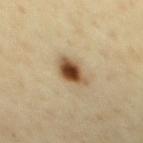<tbp_lesion>
  <biopsy_status>not biopsied; imaged during a skin examination</biopsy_status>
  <lesion_size>
    <long_diameter_mm_approx>3.5</long_diameter_mm_approx>
  </lesion_size>
  <site>chest</site>
  <lighting>cross-polarized</lighting>
  <image>
    <source>total-body photography crop</source>
    <field_of_view_mm>15</field_of_view_mm>
  </image>
  <automated_metrics>
    <area_mm2_approx>7.0</area_mm2_approx>
    <eccentricity>0.65</eccentricity>
    <shape_asymmetry>0.25</shape_asymmetry>
    <vs_skin_darker_L>19.0</vs_skin_darker_L>
    <vs_skin_contrast_norm>13.0</vs_skin_contrast_norm>
    <color_variation_0_10>6.0</color_variation_0_10>
    <peripheral_color_asymmetry>1.0</peripheral_color_asymmetry>
  </automated_metrics>
  <patient>
    <sex>male</sex>
    <age_approx>65</age_approx>
  </patient>
</tbp_lesion>